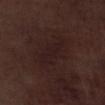Impression:
Captured during whole-body skin photography for melanoma surveillance; the lesion was not biopsied.
Context:
About 5.5 mm across. The tile uses white-light illumination. The lesion is on the left lower leg. A 15 mm close-up tile from a total-body photography series done for melanoma screening. The subject is a male aged 68–72. An algorithmic analysis of the crop reported a lesion color around L≈18 a*≈15 b*≈15 in CIELAB and roughly 4 lightness units darker than nearby skin.